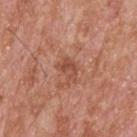Q: Was a biopsy performed?
A: imaged on a skin check; not biopsied
Q: Patient demographics?
A: male, aged 63–67
Q: Where on the body is the lesion?
A: the upper back
Q: What did automated image analysis measure?
A: a nevus-likeness score of about 0/100 and a detector confidence of about 100 out of 100 that the crop contains a lesion
Q: What lighting was used for the tile?
A: white-light illumination
Q: What is the imaging modality?
A: 15 mm crop, total-body photography
Q: What is the lesion's diameter?
A: ~3 mm (longest diameter)